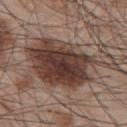  biopsy_status: not biopsied; imaged during a skin examination
  site: upper back
  patient:
    sex: male
    age_approx: 65
  image:
    source: total-body photography crop
    field_of_view_mm: 15